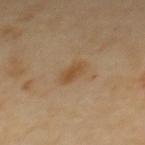Impression:
This lesion was catalogued during total-body skin photography and was not selected for biopsy.
Acquisition and patient details:
A close-up tile cropped from a whole-body skin photograph, about 15 mm across. The subject is a female approximately 60 years of age. The lesion is on the upper back.